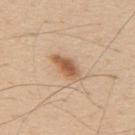notes = imaged on a skin check; not biopsied | location = the back | image = 15 mm crop, total-body photography | patient = male, aged around 60 | illumination = white-light illumination.